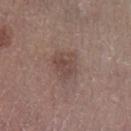The lesion was tiled from a total-body skin photograph and was not biopsied. Located on the leg. This is a white-light tile. A close-up tile cropped from a whole-body skin photograph, about 15 mm across. An algorithmic analysis of the crop reported an area of roughly 7 mm², a shape eccentricity near 0.55, and two-axis asymmetry of about 0.3. The software also gave an average lesion color of about L≈45 a*≈17 b*≈22 (CIELAB), a lesion–skin lightness drop of about 7, and a normalized border contrast of about 6. Approximately 3 mm at its widest. A male subject aged 73–77.